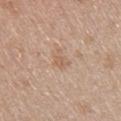Q: Is there a histopathology result?
A: total-body-photography surveillance lesion; no biopsy
Q: What is the lesion's diameter?
A: about 3 mm
Q: What kind of image is this?
A: ~15 mm crop, total-body skin-cancer survey
Q: What are the patient's age and sex?
A: female, aged around 50
Q: Lesion location?
A: the right upper arm